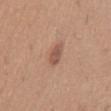The lesion was photographed on a routine skin check and not biopsied; there is no pathology result. Located on the abdomen. The total-body-photography lesion software estimated a lesion area of about 4 mm², an eccentricity of roughly 0.85, and a symmetry-axis asymmetry near 0.2. The software also gave a mean CIELAB color near L≈53 a*≈21 b*≈28 and about 10 CIELAB-L* units darker than the surrounding skin. And it measured border irregularity of about 2 on a 0–10 scale and a peripheral color-asymmetry measure near 1. The software also gave an automated nevus-likeness rating near 50 out of 100 and a detector confidence of about 100 out of 100 that the crop contains a lesion. Captured under white-light illumination. Measured at roughly 3 mm in maximum diameter. Cropped from a total-body skin-imaging series; the visible field is about 15 mm. The patient is a male aged around 60.